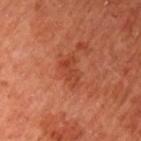{
  "biopsy_status": "not biopsied; imaged during a skin examination",
  "lesion_size": {
    "long_diameter_mm_approx": 3.5
  },
  "lighting": "cross-polarized",
  "patient": {
    "sex": "female",
    "age_approx": 65
  },
  "image": {
    "source": "total-body photography crop",
    "field_of_view_mm": 15
  },
  "site": "left upper arm"
}